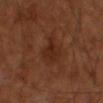- notes · total-body-photography surveillance lesion; no biopsy
- image source · ~15 mm tile from a whole-body skin photo
- TBP lesion metrics · a border-irregularity rating of about 3.5/10, a color-variation rating of about 2.5/10, and a peripheral color-asymmetry measure near 1; an automated nevus-likeness rating near 5 out of 100
- subject · male, aged approximately 65
- tile lighting · cross-polarized illumination
- diameter · about 4 mm